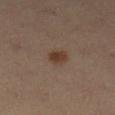biopsy status — catalogued during a skin exam; not biopsied
subject — female, about 35 years old
tile lighting — cross-polarized
acquisition — ~15 mm tile from a whole-body skin photo
automated lesion analysis — an area of roughly 4 mm² and a shape-asymmetry score of about 0.2 (0 = symmetric); an average lesion color of about L≈37 a*≈16 b*≈25 (CIELAB) and about 9 CIELAB-L* units darker than the surrounding skin; a border-irregularity rating of about 2/10, a within-lesion color-variation index near 2.5/10, and radial color variation of about 1
site — the left lower leg